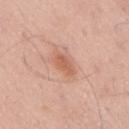Case summary:
– biopsy status: imaged on a skin check; not biopsied
– anatomic site: the mid back
– acquisition: ~15 mm tile from a whole-body skin photo
– lesion diameter: ~3 mm (longest diameter)
– patient: male, approximately 55 years of age
– automated metrics: a nevus-likeness score of about 75/100